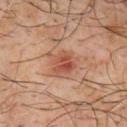Clinical impression:
This lesion was catalogued during total-body skin photography and was not selected for biopsy.
Background:
A roughly 15 mm field-of-view crop from a total-body skin photograph. The tile uses cross-polarized illumination. A male subject roughly 65 years of age. The lesion's longest dimension is about 3.5 mm. The lesion is on the upper back.